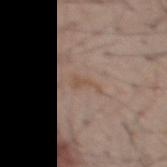Findings:
– follow-up · total-body-photography surveillance lesion; no biopsy
– acquisition · 15 mm crop, total-body photography
– patient · male, aged around 60
– diameter · ~3 mm (longest diameter)
– location · the mid back